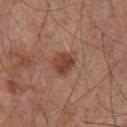Image and clinical context:
Captured under white-light illumination. About 2.5 mm across. The subject is a male approximately 65 years of age. A roughly 15 mm field-of-view crop from a total-body skin photograph. The lesion is on the front of the torso. Automated tile analysis of the lesion measured a lesion area of about 6 mm², an eccentricity of roughly 0.45, and a shape-asymmetry score of about 0.15 (0 = symmetric). The software also gave a border-irregularity index near 1.5/10, a within-lesion color-variation index near 4/10, and peripheral color asymmetry of about 1.5.